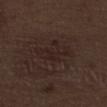biopsy status=total-body-photography surveillance lesion; no biopsy
anatomic site=the left lower leg
patient=male, in their 70s
imaging modality=total-body-photography crop, ~15 mm field of view
automated lesion analysis=a lesion–skin lightness drop of about 4 and a normalized lesion–skin contrast near 5; a nevus-likeness score of about 0/100 and a lesion-detection confidence of about 100/100